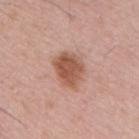Clinical impression:
Imaged during a routine full-body skin examination; the lesion was not biopsied and no histopathology is available.
Background:
Located on the mid back. A male subject aged 53–57. A roughly 15 mm field-of-view crop from a total-body skin photograph.